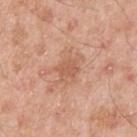Impression: The lesion was tiled from a total-body skin photograph and was not biopsied. Clinical summary: The lesion is located on the left upper arm. This image is a 15 mm lesion crop taken from a total-body photograph. A male patient approximately 55 years of age. Measured at roughly 2.5 mm in maximum diameter. This is a white-light tile.